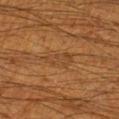workup: no biopsy performed (imaged during a skin exam)
illumination: cross-polarized
patient: male, aged 58–62
image-analysis metrics: an area of roughly 5 mm², an eccentricity of roughly 0.85, and two-axis asymmetry of about 0.3; border irregularity of about 3.5 on a 0–10 scale, a color-variation rating of about 2.5/10, and radial color variation of about 1
diameter: ≈3.5 mm
imaging modality: ~15 mm crop, total-body skin-cancer survey
anatomic site: the leg